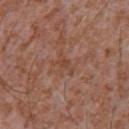  biopsy_status: not biopsied; imaged during a skin examination
  lesion_size:
    long_diameter_mm_approx: 3.0
  lighting: white-light
  site: chest
  patient:
    sex: male
    age_approx: 45
  image:
    source: total-body photography crop
    field_of_view_mm: 15